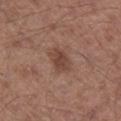Assessment:
The lesion was photographed on a routine skin check and not biopsied; there is no pathology result.
Acquisition and patient details:
Measured at roughly 3 mm in maximum diameter. Captured under white-light illumination. Cropped from a total-body skin-imaging series; the visible field is about 15 mm. Located on the right lower leg. A male subject aged 53–57.